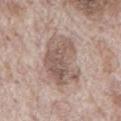This lesion was catalogued during total-body skin photography and was not selected for biopsy.
Measured at roughly 7.5 mm in maximum diameter.
This is a white-light tile.
A roughly 15 mm field-of-view crop from a total-body skin photograph.
A male subject, aged around 70.
The lesion is on the chest.